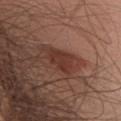biopsy_status: not biopsied; imaged during a skin examination
lighting: white-light
automated_metrics:
  area_mm2_approx: 5.5
  eccentricity: 0.55
  shape_asymmetry: 0.25
patient:
  sex: male
  age_approx: 55
site: upper back
image:
  source: total-body photography crop
  field_of_view_mm: 15